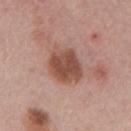follow-up: imaged on a skin check; not biopsied
anatomic site: the mid back
automated lesion analysis: a shape eccentricity near 0.7 and a shape-asymmetry score of about 0.2 (0 = symmetric); a mean CIELAB color near L≈50 a*≈23 b*≈27, roughly 12 lightness units darker than nearby skin, and a normalized border contrast of about 9; border irregularity of about 2 on a 0–10 scale, a color-variation rating of about 4/10, and a peripheral color-asymmetry measure near 1; a nevus-likeness score of about 50/100 and a lesion-detection confidence of about 100/100
lighting: white-light
lesion size: ≈5.5 mm
subject: male, aged 53 to 57
imaging modality: ~15 mm tile from a whole-body skin photo